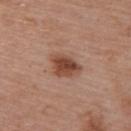Q: Is there a histopathology result?
A: imaged on a skin check; not biopsied
Q: Lesion location?
A: the upper back
Q: What is the lesion's diameter?
A: about 4 mm
Q: Who is the patient?
A: female, about 60 years old
Q: What is the imaging modality?
A: 15 mm crop, total-body photography
Q: What lighting was used for the tile?
A: white-light